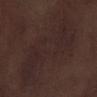biopsy status: total-body-photography surveillance lesion; no biopsy | site: the right lower leg | image: ~15 mm crop, total-body skin-cancer survey | tile lighting: white-light illumination | subject: male, aged approximately 70 | automated metrics: an area of roughly 49 mm², a shape eccentricity near 0.85, and a shape-asymmetry score of about 0.45 (0 = symmetric); a lesion color around L≈24 a*≈14 b*≈15 in CIELAB, roughly 3 lightness units darker than nearby skin, and a lesion-to-skin contrast of about 4.5 (normalized; higher = more distinct); a border-irregularity index near 7.5/10, a within-lesion color-variation index near 3/10, and radial color variation of about 1 | lesion size: ≈11.5 mm.